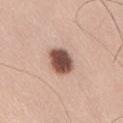Captured during whole-body skin photography for melanoma surveillance; the lesion was not biopsied. Imaged with white-light lighting. The recorded lesion diameter is about 3.5 mm. A male patient, aged 33 to 37. The lesion-visualizer software estimated an eccentricity of roughly 0.5. The analysis additionally found a mean CIELAB color near L≈51 a*≈20 b*≈25, about 21 CIELAB-L* units darker than the surrounding skin, and a normalized lesion–skin contrast near 13. A 15 mm close-up extracted from a 3D total-body photography capture. The lesion is located on the lower back.